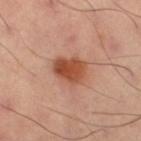biopsy_status: not biopsied; imaged during a skin examination
lesion_size:
  long_diameter_mm_approx: 4.0
site: right thigh
image:
  source: total-body photography crop
  field_of_view_mm: 15
automated_metrics:
  cielab_L: 51
  cielab_a: 27
  cielab_b: 34
  nevus_likeness_0_100: 100
  lesion_detection_confidence_0_100: 100